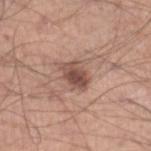Captured during whole-body skin photography for melanoma surveillance; the lesion was not biopsied.
The subject is a male aged approximately 60.
Imaged with white-light lighting.
Approximately 3.5 mm at its widest.
Located on the leg.
A 15 mm crop from a total-body photograph taken for skin-cancer surveillance.